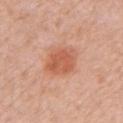This lesion was catalogued during total-body skin photography and was not selected for biopsy.